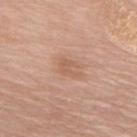Findings:
- workup — catalogued during a skin exam; not biopsied
- subject — female, roughly 70 years of age
- image — 15 mm crop, total-body photography
- location — the back